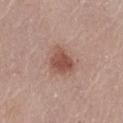Q: Was this lesion biopsied?
A: total-body-photography surveillance lesion; no biopsy
Q: Patient demographics?
A: female, aged 63 to 67
Q: Lesion location?
A: the abdomen
Q: What is the imaging modality?
A: ~15 mm crop, total-body skin-cancer survey